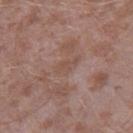This lesion was catalogued during total-body skin photography and was not selected for biopsy.
The subject is a male aged around 45.
Cropped from a total-body skin-imaging series; the visible field is about 15 mm.
From the right thigh.
An algorithmic analysis of the crop reported a lesion area of about 3.5 mm² and an outline eccentricity of about 0.9 (0 = round, 1 = elongated). And it measured about 5 CIELAB-L* units darker than the surrounding skin and a normalized border contrast of about 4.5. The software also gave border irregularity of about 4.5 on a 0–10 scale, a within-lesion color-variation index near 0.5/10, and a peripheral color-asymmetry measure near 0.5. The analysis additionally found a classifier nevus-likeness of about 0/100 and lesion-presence confidence of about 85/100.